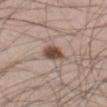{
  "biopsy_status": "not biopsied; imaged during a skin examination",
  "patient": {
    "sex": "male",
    "age_approx": 30
  },
  "image": {
    "source": "total-body photography crop",
    "field_of_view_mm": 15
  },
  "site": "leg",
  "lighting": "white-light",
  "automated_metrics": {
    "area_mm2_approx": 6.0,
    "shape_asymmetry": 0.25
  }
}